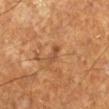image source=15 mm crop, total-body photography | patient=male, aged 58–62 | anatomic site=the left lower leg.